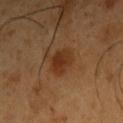biopsy status = imaged on a skin check; not biopsied
subject = male, in their 50s
anatomic site = the right upper arm
tile lighting = cross-polarized illumination
lesion size = ≈3 mm
image source = total-body-photography crop, ~15 mm field of view